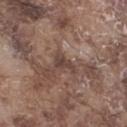notes = total-body-photography surveillance lesion; no biopsy
image source = 15 mm crop, total-body photography
image-analysis metrics = a detector confidence of about 65 out of 100 that the crop contains a lesion
illumination = white-light
patient = male, in their mid-70s
anatomic site = the leg
size = ≈3 mm